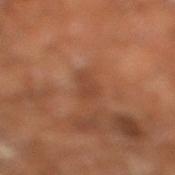workup — catalogued during a skin exam; not biopsied
location — the leg
imaging modality — ~15 mm tile from a whole-body skin photo
subject — male, approximately 60 years of age